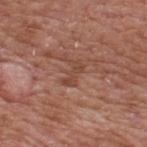Captured during whole-body skin photography for melanoma surveillance; the lesion was not biopsied.
The lesion's longest dimension is about 3 mm.
This image is a 15 mm lesion crop taken from a total-body photograph.
The tile uses white-light illumination.
From the upper back.
A male subject, approximately 65 years of age.
The total-body-photography lesion software estimated a footprint of about 4 mm², a shape eccentricity near 0.85, and a symmetry-axis asymmetry near 0.3. The software also gave a lesion color around L≈45 a*≈23 b*≈28 in CIELAB, a lesion–skin lightness drop of about 7, and a normalized border contrast of about 5.5. It also reported border irregularity of about 5.5 on a 0–10 scale, a color-variation rating of about 1/10, and peripheral color asymmetry of about 0.5.